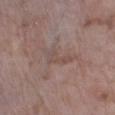The lesion was tiled from a total-body skin photograph and was not biopsied. The tile uses white-light illumination. A 15 mm close-up tile from a total-body photography series done for melanoma screening. On the left lower leg. The recorded lesion diameter is about 3.5 mm. A female patient aged 73 to 77.